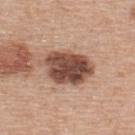workup: imaged on a skin check; not biopsied | size: ≈6.5 mm | illumination: white-light illumination | image: ~15 mm crop, total-body skin-cancer survey | body site: the upper back | TBP lesion metrics: a footprint of about 18 mm², a shape eccentricity near 0.8, and a shape-asymmetry score of about 0.15 (0 = symmetric); an average lesion color of about L≈48 a*≈21 b*≈27 (CIELAB), about 19 CIELAB-L* units darker than the surrounding skin, and a normalized lesion–skin contrast near 12.5; a border-irregularity rating of about 2/10, a color-variation rating of about 6/10, and radial color variation of about 2; an automated nevus-likeness rating near 70 out of 100 and a lesion-detection confidence of about 100/100 | patient: male, approximately 55 years of age.